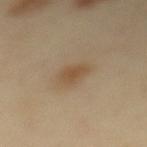workup — total-body-photography surveillance lesion; no biopsy
image source — ~15 mm tile from a whole-body skin photo
automated lesion analysis — an area of roughly 5.5 mm², an eccentricity of roughly 0.85, and a symmetry-axis asymmetry near 0.3; an automated nevus-likeness rating near 55 out of 100 and lesion-presence confidence of about 100/100
anatomic site — the mid back
subject — female, approximately 40 years of age
lesion size — about 3.5 mm